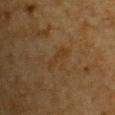Clinical impression:
This lesion was catalogued during total-body skin photography and was not selected for biopsy.
Context:
Longest diameter approximately 3 mm. A male patient, about 85 years old or older. Captured under cross-polarized illumination. The lesion is located on the front of the torso. A close-up tile cropped from a whole-body skin photograph, about 15 mm across.